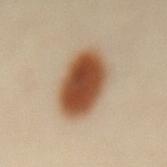<tbp_lesion>
<biopsy_status>not biopsied; imaged during a skin examination</biopsy_status>
<patient>
  <sex>female</sex>
  <age_approx>40</age_approx>
</patient>
<image>
  <source>total-body photography crop</source>
  <field_of_view_mm>15</field_of_view_mm>
</image>
<site>mid back</site>
</tbp_lesion>